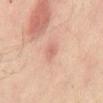notes = imaged on a skin check; not biopsied | site = the back | patient = male, aged around 50 | size = ~2.5 mm (longest diameter) | image = total-body-photography crop, ~15 mm field of view | automated lesion analysis = a nevus-likeness score of about 0/100 and a lesion-detection confidence of about 100/100.